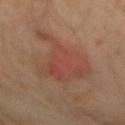Assessment:
The lesion was tiled from a total-body skin photograph and was not biopsied.
Context:
Measured at roughly 7 mm in maximum diameter. The lesion is on the mid back. A close-up tile cropped from a whole-body skin photograph, about 15 mm across. The subject is a male in their 30s.